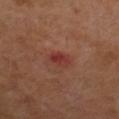No biopsy was performed on this lesion — it was imaged during a full skin examination and was not determined to be concerning. Automated image analysis of the tile measured a lesion area of about 4.5 mm², an eccentricity of roughly 0.7, and two-axis asymmetry of about 0.2. The software also gave a lesion color around L≈37 a*≈28 b*≈27 in CIELAB and about 8 CIELAB-L* units darker than the surrounding skin. It also reported border irregularity of about 1.5 on a 0–10 scale, internal color variation of about 4.5 on a 0–10 scale, and radial color variation of about 1.5. And it measured a classifier nevus-likeness of about 10/100 and a detector confidence of about 100 out of 100 that the crop contains a lesion. The patient is a female aged 53 to 57. On the left upper arm. Cropped from a total-body skin-imaging series; the visible field is about 15 mm. Captured under cross-polarized illumination. Longest diameter approximately 2.5 mm.